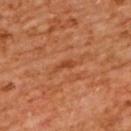biopsy status: no biopsy performed (imaged during a skin exam)
site: the back
imaging modality: 15 mm crop, total-body photography
lesion diameter: ~2.5 mm (longest diameter)
patient: female, approximately 55 years of age
lighting: cross-polarized